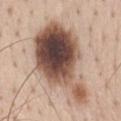Q: Was this lesion biopsied?
A: catalogued during a skin exam; not biopsied
Q: How was the tile lit?
A: white-light
Q: Where on the body is the lesion?
A: the front of the torso
Q: What are the patient's age and sex?
A: male, aged 43–47
Q: What did automated image analysis measure?
A: a lesion color around L≈51 a*≈17 b*≈25 in CIELAB and a lesion-to-skin contrast of about 13.5 (normalized; higher = more distinct); a classifier nevus-likeness of about 95/100 and a detector confidence of about 100 out of 100 that the crop contains a lesion
Q: What is the lesion's diameter?
A: about 11.5 mm
Q: What is the imaging modality?
A: ~15 mm tile from a whole-body skin photo